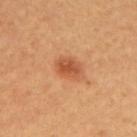Captured during whole-body skin photography for melanoma surveillance; the lesion was not biopsied.
A female subject.
Longest diameter approximately 3.5 mm.
The lesion is located on the upper back.
Cropped from a whole-body photographic skin survey; the tile spans about 15 mm.